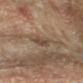Imaged during a routine full-body skin examination; the lesion was not biopsied and no histopathology is available. The patient is female. Located on the right forearm. Approximately 3.5 mm at its widest. This image is a 15 mm lesion crop taken from a total-body photograph.